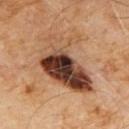A region of skin cropped from a whole-body photographic capture, roughly 15 mm wide. Longest diameter approximately 8 mm. From the chest. An algorithmic analysis of the crop reported an area of roughly 27 mm², an outline eccentricity of about 0.8 (0 = round, 1 = elongated), and a symmetry-axis asymmetry near 0.4. It also reported a border-irregularity index near 6/10 and a color-variation rating of about 10/10. The software also gave lesion-presence confidence of about 100/100. This is a cross-polarized tile. The patient is a male aged around 60.